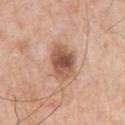| field | value |
|---|---|
| notes | total-body-photography surveillance lesion; no biopsy |
| lesion diameter | about 4.5 mm |
| subject | male, in their 70s |
| illumination | white-light |
| image | ~15 mm tile from a whole-body skin photo |
| anatomic site | the left upper arm |